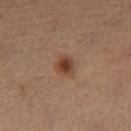Q: Was this lesion biopsied?
A: no biopsy performed (imaged during a skin exam)
Q: How was this image acquired?
A: ~15 mm crop, total-body skin-cancer survey
Q: Lesion size?
A: about 2.5 mm
Q: How was the tile lit?
A: cross-polarized
Q: What is the anatomic site?
A: the left leg
Q: Patient demographics?
A: male, about 50 years old